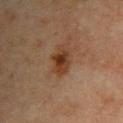No biopsy was performed on this lesion — it was imaged during a full skin examination and was not determined to be concerning.
Approximately 3.5 mm at its widest.
From the upper back.
Captured under cross-polarized illumination.
Cropped from a whole-body photographic skin survey; the tile spans about 15 mm.
A male patient, about 45 years old.